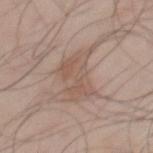biopsy_status: not biopsied; imaged during a skin examination
site: mid back
image:
  source: total-body photography crop
  field_of_view_mm: 15
patient:
  sex: male
  age_approx: 45
lesion_size:
  long_diameter_mm_approx: 6.0
lighting: white-light